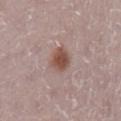{
  "biopsy_status": "not biopsied; imaged during a skin examination",
  "site": "leg",
  "patient": {
    "sex": "female",
    "age_approx": 30
  },
  "lesion_size": {
    "long_diameter_mm_approx": 3.0
  },
  "image": {
    "source": "total-body photography crop",
    "field_of_view_mm": 15
  }
}